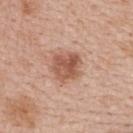Impression: The lesion was tiled from a total-body skin photograph and was not biopsied. Clinical summary: Captured under white-light illumination. Automated tile analysis of the lesion measured a shape eccentricity near 0.3. And it measured border irregularity of about 2 on a 0–10 scale, a color-variation rating of about 5/10, and a peripheral color-asymmetry measure near 2. The analysis additionally found lesion-presence confidence of about 100/100. On the upper back. Cropped from a total-body skin-imaging series; the visible field is about 15 mm. Measured at roughly 3.5 mm in maximum diameter. The patient is a female about 50 years old.